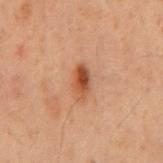Imaged during a routine full-body skin examination; the lesion was not biopsied and no histopathology is available.